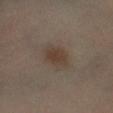Impression:
The lesion was tiled from a total-body skin photograph and was not biopsied.
Clinical summary:
A roughly 15 mm field-of-view crop from a total-body skin photograph. The patient is a male approximately 50 years of age. The lesion is on the left lower leg. The recorded lesion diameter is about 3.5 mm. Captured under cross-polarized illumination.